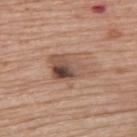Imaged during a routine full-body skin examination; the lesion was not biopsied and no histopathology is available. Located on the upper back. Imaged with white-light lighting. About 5.5 mm across. Cropped from a total-body skin-imaging series; the visible field is about 15 mm. A female patient, aged 63 to 67.